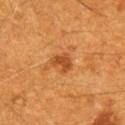Findings:
* notes — imaged on a skin check; not biopsied
* subject — male, aged approximately 60
* site — the back
* imaging modality — total-body-photography crop, ~15 mm field of view